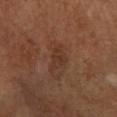Notes:
- follow-up · total-body-photography surveillance lesion; no biopsy
- automated metrics · an average lesion color of about L≈33 a*≈19 b*≈26 (CIELAB) and about 6 CIELAB-L* units darker than the surrounding skin
- size · about 3 mm
- patient · female, aged approximately 65
- imaging modality · 15 mm crop, total-body photography
- anatomic site · the right lower leg